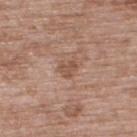Notes:
- tile lighting · white-light illumination
- patient · male, aged approximately 50
- anatomic site · the upper back
- image source · 15 mm crop, total-body photography
- diameter · ~2.5 mm (longest diameter)
- automated metrics · an average lesion color of about L≈52 a*≈19 b*≈28 (CIELAB), roughly 8 lightness units darker than nearby skin, and a normalized lesion–skin contrast near 6; a color-variation rating of about 1.5/10; an automated nevus-likeness rating near 0 out of 100 and a detector confidence of about 100 out of 100 that the crop contains a lesion